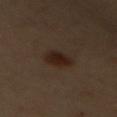TBP lesion metrics=a border-irregularity rating of about 2.5/10, internal color variation of about 3.5 on a 0–10 scale, and radial color variation of about 1.5; a nevus-likeness score of about 90/100 and a detector confidence of about 100 out of 100 that the crop contains a lesion
subject=female, roughly 60 years of age
site=the mid back
image source=~15 mm tile from a whole-body skin photo